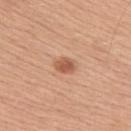Q: Was a biopsy performed?
A: catalogued during a skin exam; not biopsied